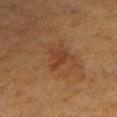No biopsy was performed on this lesion — it was imaged during a full skin examination and was not determined to be concerning. A female patient in their mid-50s. Captured under cross-polarized illumination. The lesion is on the right forearm. A 15 mm close-up extracted from a 3D total-body photography capture. Longest diameter approximately 3 mm.